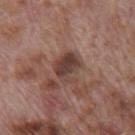Assessment: The lesion was tiled from a total-body skin photograph and was not biopsied.